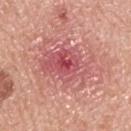Notes:
– notes · catalogued during a skin exam; not biopsied
– lighting · white-light illumination
– subject · male, in their mid-60s
– diameter · ~7 mm (longest diameter)
– image source · total-body-photography crop, ~15 mm field of view
– site · the back